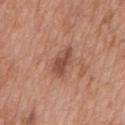Impression:
Imaged during a routine full-body skin examination; the lesion was not biopsied and no histopathology is available.